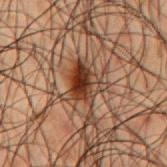biopsy_status: not biopsied; imaged during a skin examination
image:
  source: total-body photography crop
  field_of_view_mm: 15
lesion_size:
  long_diameter_mm_approx: 4.0
lighting: cross-polarized
site: back
patient:
  sex: male
  age_approx: 50